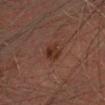Q: Is there a histopathology result?
A: catalogued during a skin exam; not biopsied